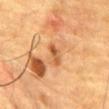{"biopsy_status": "not biopsied; imaged during a skin examination", "image": {"source": "total-body photography crop", "field_of_view_mm": 15}, "site": "chest", "patient": {"sex": "male", "age_approx": "85+"}}